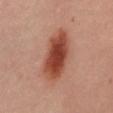{
  "biopsy_status": "not biopsied; imaged during a skin examination",
  "image": {
    "source": "total-body photography crop",
    "field_of_view_mm": 15
  },
  "lesion_size": {
    "long_diameter_mm_approx": 7.5
  },
  "patient": {
    "sex": "female",
    "age_approx": 45
  },
  "site": "mid back",
  "lighting": "cross-polarized"
}